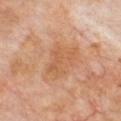lesion diameter: ≈4.5 mm
body site: the chest
tile lighting: cross-polarized
imaging modality: ~15 mm crop, total-body skin-cancer survey
patient: male, aged 68–72
TBP lesion metrics: a footprint of about 7 mm² and a symmetry-axis asymmetry near 0.45; an average lesion color of about L≈56 a*≈22 b*≈36 (CIELAB), about 6 CIELAB-L* units darker than the surrounding skin, and a normalized lesion–skin contrast near 5; a detector confidence of about 100 out of 100 that the crop contains a lesion